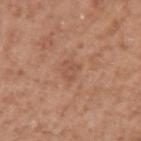biopsy_status: not biopsied; imaged during a skin examination
patient:
  sex: male
  age_approx: 50
image:
  source: total-body photography crop
  field_of_view_mm: 15
lighting: white-light
site: arm
lesion_size:
  long_diameter_mm_approx: 3.0